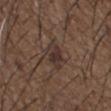Clinical impression:
The lesion was tiled from a total-body skin photograph and was not biopsied.
Background:
The patient is a male aged around 50. This image is a 15 mm lesion crop taken from a total-body photograph. Approximately 3.5 mm at its widest. On the upper back. Imaged with white-light lighting.